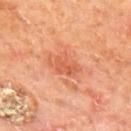Q: Was this lesion biopsied?
A: imaged on a skin check; not biopsied
Q: Who is the patient?
A: male, approximately 65 years of age
Q: What kind of image is this?
A: 15 mm crop, total-body photography
Q: What is the anatomic site?
A: the mid back